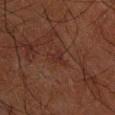Part of a total-body skin-imaging series; this lesion was reviewed on a skin check and was not flagged for biopsy.
Captured under cross-polarized illumination.
A male patient, aged 58–62.
Located on the right forearm.
Longest diameter approximately 2.5 mm.
A 15 mm close-up tile from a total-body photography series done for melanoma screening.